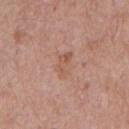| field | value |
|---|---|
| biopsy status | catalogued during a skin exam; not biopsied |
| patient | male, approximately 70 years of age |
| body site | the chest |
| image-analysis metrics | a mean CIELAB color near L≈56 a*≈21 b*≈29, roughly 6 lightness units darker than nearby skin, and a normalized lesion–skin contrast near 5; border irregularity of about 3 on a 0–10 scale, internal color variation of about 4 on a 0–10 scale, and a peripheral color-asymmetry measure near 1.5 |
| lesion size | ~3 mm (longest diameter) |
| illumination | white-light illumination |
| image | total-body-photography crop, ~15 mm field of view |